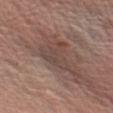| field | value |
|---|---|
| biopsy status | imaged on a skin check; not biopsied |